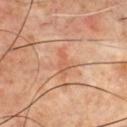Impression:
Part of a total-body skin-imaging series; this lesion was reviewed on a skin check and was not flagged for biopsy.
Clinical summary:
A male patient, aged 68–72. The lesion-visualizer software estimated a lesion color around L≈56 a*≈25 b*≈33 in CIELAB, about 7 CIELAB-L* units darker than the surrounding skin, and a lesion-to-skin contrast of about 5 (normalized; higher = more distinct). The analysis additionally found an automated nevus-likeness rating near 0 out of 100 and lesion-presence confidence of about 55/100. The tile uses cross-polarized illumination. A lesion tile, about 15 mm wide, cut from a 3D total-body photograph. From the chest. Approximately 3.5 mm at its widest.